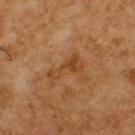This lesion was catalogued during total-body skin photography and was not selected for biopsy. Measured at roughly 4 mm in maximum diameter. A male subject aged 58 to 62. The total-body-photography lesion software estimated a border-irregularity index near 7/10 and peripheral color asymmetry of about 0.5. And it measured lesion-presence confidence of about 100/100. Located on the upper back. Imaged with cross-polarized lighting. This image is a 15 mm lesion crop taken from a total-body photograph.